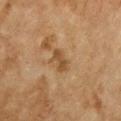Notes:
• notes — imaged on a skin check; not biopsied
• subject — female, in their 60s
• lighting — cross-polarized illumination
• diameter — about 3 mm
• imaging modality — ~15 mm crop, total-body skin-cancer survey
• anatomic site — the chest